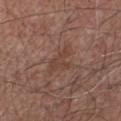Recorded during total-body skin imaging; not selected for excision or biopsy. Imaged with white-light lighting. A region of skin cropped from a whole-body photographic capture, roughly 15 mm wide. An algorithmic analysis of the crop reported a shape eccentricity near 0.85 and a shape-asymmetry score of about 0.5 (0 = symmetric). The software also gave border irregularity of about 6.5 on a 0–10 scale, a within-lesion color-variation index near 2/10, and radial color variation of about 0.5. The software also gave a classifier nevus-likeness of about 0/100 and a lesion-detection confidence of about 90/100. Located on the chest. A male subject, aged around 55.